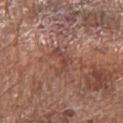The recorded lesion diameter is about 3.5 mm. This image is a 15 mm lesion crop taken from a total-body photograph. A male subject, roughly 70 years of age. On the left forearm. Automated image analysis of the tile measured an eccentricity of roughly 0.85 and a shape-asymmetry score of about 0.7 (0 = symmetric). The analysis additionally found a mean CIELAB color near L≈45 a*≈22 b*≈27, about 8 CIELAB-L* units darker than the surrounding skin, and a normalized lesion–skin contrast near 6. The software also gave border irregularity of about 9 on a 0–10 scale and a within-lesion color-variation index near 0/10.